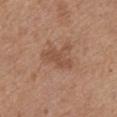Clinical summary: A female subject roughly 65 years of age. A 15 mm close-up extracted from a 3D total-body photography capture. Measured at roughly 4 mm in maximum diameter. Located on the front of the torso.